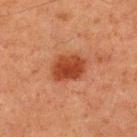Clinical impression: Captured during whole-body skin photography for melanoma surveillance; the lesion was not biopsied. Acquisition and patient details: A close-up tile cropped from a whole-body skin photograph, about 15 mm across. The subject is a male roughly 60 years of age. The lesion-visualizer software estimated a mean CIELAB color near L≈35 a*≈26 b*≈31, a lesion–skin lightness drop of about 11, and a normalized lesion–skin contrast near 9.5. Located on the upper back. Imaged with cross-polarized lighting.